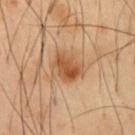A male patient, aged 53 to 57.
About 4 mm across.
A roughly 15 mm field-of-view crop from a total-body skin photograph.
Located on the chest.
This is a cross-polarized tile.